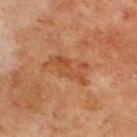notes = no biopsy performed (imaged during a skin exam) | image-analysis metrics = a lesion color around L≈50 a*≈26 b*≈38 in CIELAB and a normalized lesion–skin contrast near 5.5; a border-irregularity index near 6/10 and internal color variation of about 4.5 on a 0–10 scale; an automated nevus-likeness rating near 0 out of 100 | image source = 15 mm crop, total-body photography | lesion size = about 5.5 mm | body site = the upper back | illumination = cross-polarized | patient = male, roughly 70 years of age.